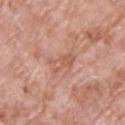No biopsy was performed on this lesion — it was imaged during a full skin examination and was not determined to be concerning.
The tile uses white-light illumination.
The patient is a male aged around 70.
Approximately 3.5 mm at its widest.
The lesion is located on the back.
A roughly 15 mm field-of-view crop from a total-body skin photograph.
An algorithmic analysis of the crop reported an average lesion color of about L≈58 a*≈24 b*≈30 (CIELAB), about 7 CIELAB-L* units darker than the surrounding skin, and a normalized lesion–skin contrast near 5.5. And it measured border irregularity of about 5 on a 0–10 scale, a color-variation rating of about 2/10, and radial color variation of about 0.5.